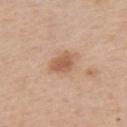Q: Was a biopsy performed?
A: catalogued during a skin exam; not biopsied
Q: What is the lesion's diameter?
A: ~3 mm (longest diameter)
Q: What is the anatomic site?
A: the left upper arm
Q: Illumination type?
A: white-light illumination
Q: What is the imaging modality?
A: ~15 mm crop, total-body skin-cancer survey
Q: Patient demographics?
A: male, aged 58 to 62